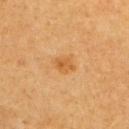Recorded during total-body skin imaging; not selected for excision or biopsy. A female patient, about 55 years old. Longest diameter approximately 2.5 mm. A region of skin cropped from a whole-body photographic capture, roughly 15 mm wide. Located on the right upper arm.